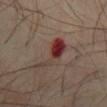{
  "biopsy_status": "not biopsied; imaged during a skin examination",
  "patient": {
    "sex": "male",
    "age_approx": 70
  },
  "image": {
    "source": "total-body photography crop",
    "field_of_view_mm": 15
  },
  "lighting": "cross-polarized",
  "site": "abdomen",
  "lesion_size": {
    "long_diameter_mm_approx": 5.5
  }
}